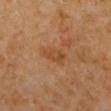The lesion was photographed on a routine skin check and not biopsied; there is no pathology result. The patient is a male about 70 years old. The lesion-visualizer software estimated a lesion area of about 5 mm². The analysis additionally found a border-irregularity rating of about 3/10, a color-variation rating of about 2.5/10, and radial color variation of about 1. It also reported a lesion-detection confidence of about 100/100. This is a cross-polarized tile. Cropped from a whole-body photographic skin survey; the tile spans about 15 mm. From the head or neck.